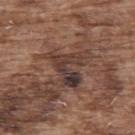This lesion was catalogued during total-body skin photography and was not selected for biopsy. The total-body-photography lesion software estimated a mean CIELAB color near L≈37 a*≈17 b*≈21, roughly 9 lightness units darker than nearby skin, and a lesion-to-skin contrast of about 9 (normalized; higher = more distinct). The analysis additionally found a nevus-likeness score of about 0/100 and a detector confidence of about 85 out of 100 that the crop contains a lesion. This is a white-light tile. The lesion is on the upper back. A male subject, about 75 years old. A lesion tile, about 15 mm wide, cut from a 3D total-body photograph.